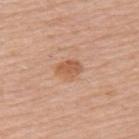Background: Measured at roughly 3 mm in maximum diameter. Located on the upper back. The tile uses white-light illumination. The patient is a female approximately 50 years of age. A close-up tile cropped from a whole-body skin photograph, about 15 mm across. An algorithmic analysis of the crop reported a border-irregularity rating of about 1.5/10, a color-variation rating of about 1.5/10, and peripheral color asymmetry of about 0.5. The analysis additionally found an automated nevus-likeness rating near 70 out of 100.